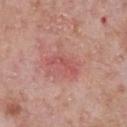{"biopsy_status": "not biopsied; imaged during a skin examination", "site": "chest", "lesion_size": {"long_diameter_mm_approx": 4.0}, "patient": {"sex": "male", "age_approx": 80}, "automated_metrics": {"cielab_L": 54, "cielab_a": 31, "cielab_b": 25, "vs_skin_darker_L": 8.0, "vs_skin_contrast_norm": 5.5, "nevus_likeness_0_100": 0, "lesion_detection_confidence_0_100": 100}, "image": {"source": "total-body photography crop", "field_of_view_mm": 15}}